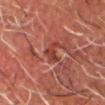body site = the head or neck; subject = male, approximately 70 years of age; image source = ~15 mm tile from a whole-body skin photo.